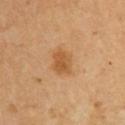biopsy_status: not biopsied; imaged during a skin examination
lesion_size:
  long_diameter_mm_approx: 3.0
site: upper back
lighting: cross-polarized
image:
  source: total-body photography crop
  field_of_view_mm: 15
patient:
  sex: female
  age_approx: 65